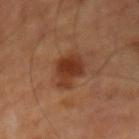• biopsy status · imaged on a skin check; not biopsied
• imaging modality · 15 mm crop, total-body photography
• illumination · cross-polarized illumination
• subject · male, aged 48 to 52
• lesion diameter · about 4 mm
• anatomic site · the left upper arm
• automated metrics · a lesion color around L≈36 a*≈24 b*≈32 in CIELAB, a lesion–skin lightness drop of about 10, and a normalized lesion–skin contrast near 9